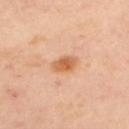Q: Is there a histopathology result?
A: no biopsy performed (imaged during a skin exam)
Q: What are the patient's age and sex?
A: female, about 40 years old
Q: Where on the body is the lesion?
A: the back
Q: How was this image acquired?
A: total-body-photography crop, ~15 mm field of view
Q: Illumination type?
A: cross-polarized
Q: How large is the lesion?
A: ≈3 mm